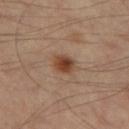workup: imaged on a skin check; not biopsied | subject: male, aged approximately 55 | lesion size: ≈2.5 mm | site: the left thigh | lighting: cross-polarized | acquisition: 15 mm crop, total-body photography.